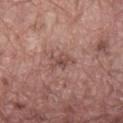Case summary:
- biopsy status · catalogued during a skin exam; not biopsied